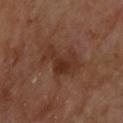Q: Was this lesion biopsied?
A: total-body-photography surveillance lesion; no biopsy
Q: How was this image acquired?
A: ~15 mm tile from a whole-body skin photo
Q: What is the anatomic site?
A: the upper back
Q: Automated lesion metrics?
A: a shape eccentricity near 0.85 and a shape-asymmetry score of about 0.5 (0 = symmetric); a lesion color around L≈31 a*≈20 b*≈26 in CIELAB, about 7 CIELAB-L* units darker than the surrounding skin, and a lesion-to-skin contrast of about 7.5 (normalized; higher = more distinct)
Q: Who is the patient?
A: male, aged 68–72
Q: How was the tile lit?
A: cross-polarized illumination